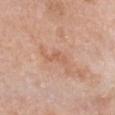  biopsy_status: not biopsied; imaged during a skin examination
  site: chest
  image:
    source: total-body photography crop
    field_of_view_mm: 15
  patient:
    sex: female
    age_approx: 75